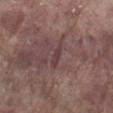<tbp_lesion>
<biopsy_status>not biopsied; imaged during a skin examination</biopsy_status>
<site>right lower leg</site>
<image>
  <source>total-body photography crop</source>
  <field_of_view_mm>15</field_of_view_mm>
</image>
<patient>
  <sex>male</sex>
  <age_approx>70</age_approx>
</patient>
<automated_metrics>
  <area_mm2_approx>2.5</area_mm2_approx>
  <shape_asymmetry>0.35</shape_asymmetry>
  <cielab_L>39</cielab_L>
  <cielab_a>20</cielab_a>
  <cielab_b>17</cielab_b>
  <vs_skin_contrast_norm>6.0</vs_skin_contrast_norm>
  <border_irregularity_0_10>3.5</border_irregularity_0_10>
  <color_variation_0_10>0.0</color_variation_0_10>
  <peripheral_color_asymmetry>0.0</peripheral_color_asymmetry>
  <nevus_likeness_0_100>0</nevus_likeness_0_100>
  <lesion_detection_confidence_0_100>55</lesion_detection_confidence_0_100>
</automated_metrics>
<lighting>white-light</lighting>
<lesion_size>
  <long_diameter_mm_approx>2.5</long_diameter_mm_approx>
</lesion_size>
</tbp_lesion>